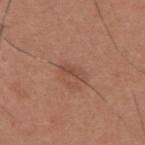biopsy status: catalogued during a skin exam; not biopsied | patient: male, aged approximately 35 | illumination: white-light | automated metrics: an automated nevus-likeness rating near 5 out of 100 and a detector confidence of about 100 out of 100 that the crop contains a lesion | lesion size: about 3 mm | image source: total-body-photography crop, ~15 mm field of view | body site: the back.